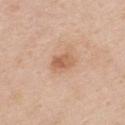{"biopsy_status": "not biopsied; imaged during a skin examination", "site": "chest", "image": {"source": "total-body photography crop", "field_of_view_mm": 15}, "patient": {"sex": "female", "age_approx": 55}}